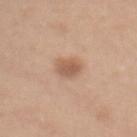biopsy status: total-body-photography surveillance lesion; no biopsy
image: 15 mm crop, total-body photography
site: the abdomen
patient: female, approximately 40 years of age